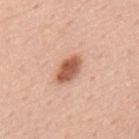Imaged during a routine full-body skin examination; the lesion was not biopsied and no histopathology is available. This image is a 15 mm lesion crop taken from a total-body photograph. On the back. A male patient, about 55 years old. Imaged with white-light lighting.